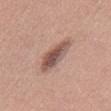- biopsy status: catalogued during a skin exam; not biopsied
- site: the chest
- image-analysis metrics: a footprint of about 10 mm², an eccentricity of roughly 0.9, and a shape-asymmetry score of about 0.25 (0 = symmetric); a mean CIELAB color near L≈53 a*≈20 b*≈24 and a lesion-to-skin contrast of about 9 (normalized; higher = more distinct); a border-irregularity index near 3/10, internal color variation of about 5.5 on a 0–10 scale, and radial color variation of about 1.5; a nevus-likeness score of about 85/100 and a detector confidence of about 100 out of 100 that the crop contains a lesion
- patient: male, aged approximately 35
- lesion diameter: ≈5.5 mm
- imaging modality: ~15 mm crop, total-body skin-cancer survey